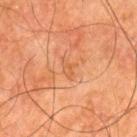workup — imaged on a skin check; not biopsied
patient — male, aged around 80
acquisition — total-body-photography crop, ~15 mm field of view
anatomic site — the left thigh
lighting — cross-polarized
diameter — ~3 mm (longest diameter)
automated lesion analysis — a footprint of about 3 mm², an eccentricity of roughly 0.8, and a symmetry-axis asymmetry near 0.45; a nevus-likeness score of about 0/100